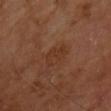notes=imaged on a skin check; not biopsied | body site=the back | patient=male, aged approximately 60 | tile lighting=cross-polarized | TBP lesion metrics=an automated nevus-likeness rating near 0 out of 100 and lesion-presence confidence of about 100/100 | image source=~15 mm crop, total-body skin-cancer survey | lesion diameter=≈3 mm.